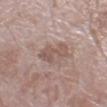  biopsy_status: not biopsied; imaged during a skin examination
  patient:
    sex: female
    age_approx: 70
  lesion_size:
    long_diameter_mm_approx: 3.5
  site: right lower leg
  image:
    source: total-body photography crop
    field_of_view_mm: 15